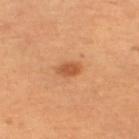The lesion was tiled from a total-body skin photograph and was not biopsied. A female subject, about 60 years old. Located on the left thigh. An algorithmic analysis of the crop reported a footprint of about 3.5 mm² and two-axis asymmetry of about 0.2. The analysis additionally found a mean CIELAB color near L≈56 a*≈28 b*≈42 and roughly 11 lightness units darker than nearby skin. It also reported a border-irregularity rating of about 2/10, internal color variation of about 1.5 on a 0–10 scale, and peripheral color asymmetry of about 0.5. A 15 mm crop from a total-body photograph taken for skin-cancer surveillance. This is a cross-polarized tile. The lesion's longest dimension is about 2.5 mm.